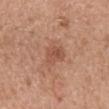Assessment: Part of a total-body skin-imaging series; this lesion was reviewed on a skin check and was not flagged for biopsy. Acquisition and patient details: The tile uses white-light illumination. A female subject aged 38 to 42. The lesion is located on the mid back. A region of skin cropped from a whole-body photographic capture, roughly 15 mm wide. The recorded lesion diameter is about 3.5 mm.